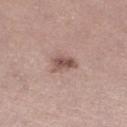Part of a total-body skin-imaging series; this lesion was reviewed on a skin check and was not flagged for biopsy.
The recorded lesion diameter is about 3 mm.
The subject is a female aged 38 to 42.
The lesion is on the right lower leg.
A 15 mm crop from a total-body photograph taken for skin-cancer surveillance.